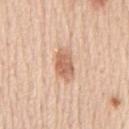biopsy status: imaged on a skin check; not biopsied
lighting: white-light illumination
subject: male, aged approximately 60
imaging modality: 15 mm crop, total-body photography
size: ≈4.5 mm
automated lesion analysis: a lesion area of about 7.5 mm², an eccentricity of roughly 0.85, and two-axis asymmetry of about 0.25; a lesion color around L≈64 a*≈22 b*≈32 in CIELAB, a lesion–skin lightness drop of about 13, and a lesion-to-skin contrast of about 7.5 (normalized; higher = more distinct); a color-variation rating of about 3.5/10 and a peripheral color-asymmetry measure near 1; an automated nevus-likeness rating near 95 out of 100
site: the back